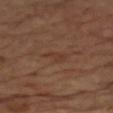The lesion was photographed on a routine skin check and not biopsied; there is no pathology result. Imaged with cross-polarized lighting. The patient is a male aged 63 to 67. The lesion's longest dimension is about 3.5 mm. A roughly 15 mm field-of-view crop from a total-body skin photograph. On the left arm. The lesion-visualizer software estimated a border-irregularity rating of about 5/10, a color-variation rating of about 0.5/10, and radial color variation of about 0.